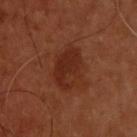Part of a total-body skin-imaging series; this lesion was reviewed on a skin check and was not flagged for biopsy. A male patient aged 53–57. This image is a 15 mm lesion crop taken from a total-body photograph. Located on the back.